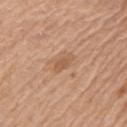Clinical impression:
The lesion was photographed on a routine skin check and not biopsied; there is no pathology result.
Clinical summary:
The patient is a male aged 68–72. Captured under white-light illumination. The lesion is located on the left upper arm. Longest diameter approximately 2.5 mm. A 15 mm crop from a total-body photograph taken for skin-cancer surveillance.